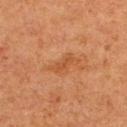{"biopsy_status": "not biopsied; imaged during a skin examination", "patient": {"sex": "female", "age_approx": 50}, "image": {"source": "total-body photography crop", "field_of_view_mm": 15}, "lighting": "cross-polarized", "site": "upper back"}